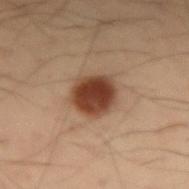No biopsy was performed on this lesion — it was imaged during a full skin examination and was not determined to be concerning. A male subject approximately 55 years of age. An algorithmic analysis of the crop reported an eccentricity of roughly 0.55 and a symmetry-axis asymmetry near 0.15. And it measured an average lesion color of about L≈33 a*≈18 b*≈25 (CIELAB), roughly 14 lightness units darker than nearby skin, and a normalized border contrast of about 12.5. And it measured a border-irregularity rating of about 1.5/10. From the right forearm. A close-up tile cropped from a whole-body skin photograph, about 15 mm across.